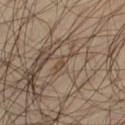biopsy_status: not biopsied; imaged during a skin examination
lesion_size:
  long_diameter_mm_approx: 3.0
lighting: cross-polarized
site: left thigh
patient:
  sex: male
  age_approx: 45
image:
  source: total-body photography crop
  field_of_view_mm: 15
automated_metrics:
  border_irregularity_0_10: 4.0
  color_variation_0_10: 0.0
  peripheral_color_asymmetry: 0.0